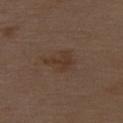workup: imaged on a skin check; not biopsied | lesion diameter: ~3.5 mm (longest diameter) | imaging modality: total-body-photography crop, ~15 mm field of view | lighting: white-light illumination | subject: male, about 70 years old | location: the upper back.